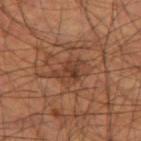Impression:
Imaged during a routine full-body skin examination; the lesion was not biopsied and no histopathology is available.
Acquisition and patient details:
On the left thigh. A roughly 15 mm field-of-view crop from a total-body skin photograph. A male patient, in their mid- to late 50s. Imaged with cross-polarized lighting. The recorded lesion diameter is about 3.5 mm.